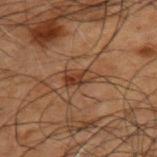patient — male, aged 48–52 | imaging modality — ~15 mm tile from a whole-body skin photo | lesion size — ≈3.5 mm | body site — the upper back | lighting — cross-polarized illumination.